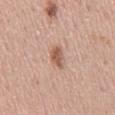Recorded during total-body skin imaging; not selected for excision or biopsy.
From the mid back.
This is a white-light tile.
The subject is a male aged around 55.
A close-up tile cropped from a whole-body skin photograph, about 15 mm across.
The recorded lesion diameter is about 3 mm.